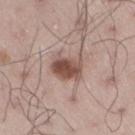Assessment: The lesion was photographed on a routine skin check and not biopsied; there is no pathology result. Image and clinical context: This is a white-light tile. A 15 mm crop from a total-body photograph taken for skin-cancer surveillance. Automated image analysis of the tile measured a lesion–skin lightness drop of about 13 and a lesion-to-skin contrast of about 9.5 (normalized; higher = more distinct). The analysis additionally found a classifier nevus-likeness of about 95/100 and lesion-presence confidence of about 100/100. About 3.5 mm across. The subject is a male aged 48–52. The lesion is located on the right thigh.